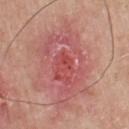{
  "diagnosis": {
    "histopathology": "squamous cell carcinoma",
    "malignancy": "malignant",
    "taxonomic_path": [
      "Malignant",
      "Malignant epidermal proliferations",
      "Squamous cell carcinoma, NOS"
    ]
  }
}